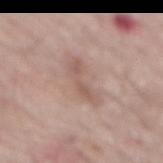No biopsy was performed on this lesion — it was imaged during a full skin examination and was not determined to be concerning. This is a white-light tile. A male patient, about 70 years old. From the back. The recorded lesion diameter is about 5 mm. Automated image analysis of the tile measured a lesion area of about 7.5 mm², an eccentricity of roughly 0.95, and two-axis asymmetry of about 0.45. The software also gave border irregularity of about 6 on a 0–10 scale, a color-variation rating of about 1.5/10, and radial color variation of about 0.5. It also reported a classifier nevus-likeness of about 0/100 and lesion-presence confidence of about 100/100. A close-up tile cropped from a whole-body skin photograph, about 15 mm across.